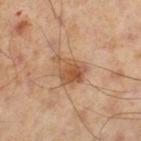Q: Was a biopsy performed?
A: imaged on a skin check; not biopsied